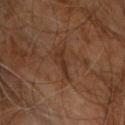Q: Was a biopsy performed?
A: catalogued during a skin exam; not biopsied
Q: What is the lesion's diameter?
A: ~3.5 mm (longest diameter)
Q: Where on the body is the lesion?
A: the chest
Q: What kind of image is this?
A: total-body-photography crop, ~15 mm field of view
Q: Patient demographics?
A: male, aged 58 to 62
Q: Illumination type?
A: cross-polarized illumination